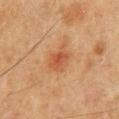This lesion was catalogued during total-body skin photography and was not selected for biopsy. The subject is a male about 50 years old. Approximately 4 mm at its widest. On the front of the torso. A 15 mm close-up extracted from a 3D total-body photography capture.